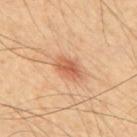This lesion was catalogued during total-body skin photography and was not selected for biopsy. This is a cross-polarized tile. The total-body-photography lesion software estimated an average lesion color of about L≈60 a*≈25 b*≈36 (CIELAB) and a lesion-to-skin contrast of about 7 (normalized; higher = more distinct). A lesion tile, about 15 mm wide, cut from a 3D total-body photograph. The subject is a male in their mid-60s. Located on the mid back.